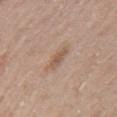Case summary:
• notes — total-body-photography surveillance lesion; no biopsy
• diameter — ≈3 mm
• anatomic site — the left upper arm
• subject — female, aged around 60
• TBP lesion metrics — a lesion-detection confidence of about 100/100
• imaging modality — ~15 mm tile from a whole-body skin photo
• lighting — white-light